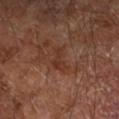{"biopsy_status": "not biopsied; imaged during a skin examination", "image": {"source": "total-body photography crop", "field_of_view_mm": 15}, "site": "right forearm", "lesion_size": {"long_diameter_mm_approx": 3.5}, "patient": {"sex": "male", "age_approx": 65}, "lighting": "cross-polarized", "automated_metrics": {"area_mm2_approx": 5.0, "eccentricity": 0.85, "cielab_L": 32, "cielab_a": 20, "cielab_b": 26, "vs_skin_contrast_norm": 6.0, "border_irregularity_0_10": 5.5, "peripheral_color_asymmetry": 0.5}}